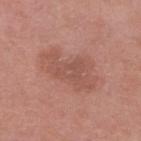follow-up: imaged on a skin check; not biopsied | lighting: white-light | patient: male, approximately 85 years of age or older | acquisition: total-body-photography crop, ~15 mm field of view | TBP lesion metrics: a lesion color around L≈53 a*≈24 b*≈27 in CIELAB, roughly 7 lightness units darker than nearby skin, and a lesion-to-skin contrast of about 5 (normalized; higher = more distinct); a nevus-likeness score of about 0/100 | location: the right lower leg.